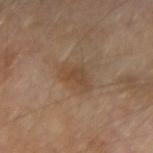No biopsy was performed on this lesion — it was imaged during a full skin examination and was not determined to be concerning.